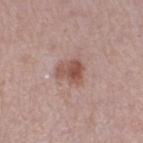The lesion was photographed on a routine skin check and not biopsied; there is no pathology result.
A female subject about 30 years old.
A lesion tile, about 15 mm wide, cut from a 3D total-body photograph.
On the right thigh.
The recorded lesion diameter is about 3.5 mm.